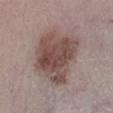Q: Was this lesion biopsied?
A: total-body-photography surveillance lesion; no biopsy
Q: Where on the body is the lesion?
A: the left lower leg
Q: Lesion size?
A: ~7.5 mm (longest diameter)
Q: Illumination type?
A: white-light
Q: What is the imaging modality?
A: total-body-photography crop, ~15 mm field of view
Q: What are the patient's age and sex?
A: male, about 80 years old